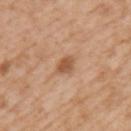biopsy_status: not biopsied; imaged during a skin examination
patient:
  sex: male
  age_approx: 65
image:
  source: total-body photography crop
  field_of_view_mm: 15
automated_metrics:
  nevus_likeness_0_100: 75
  lesion_detection_confidence_0_100: 100
lesion_size:
  long_diameter_mm_approx: 3.0
site: left upper arm
lighting: white-light